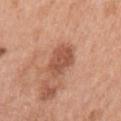Assessment:
Recorded during total-body skin imaging; not selected for excision or biopsy.
Background:
The lesion-visualizer software estimated a lesion area of about 8.5 mm². On the left upper arm. Cropped from a whole-body photographic skin survey; the tile spans about 15 mm. A female subject aged 38–42. The tile uses white-light illumination.